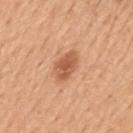Case summary:
* biopsy status — catalogued during a skin exam; not biopsied
* location — the mid back
* image — ~15 mm tile from a whole-body skin photo
* size — ~4 mm (longest diameter)
* automated metrics — an area of roughly 7 mm² and two-axis asymmetry of about 0.2; border irregularity of about 2 on a 0–10 scale, a color-variation rating of about 3/10, and peripheral color asymmetry of about 1; a detector confidence of about 100 out of 100 that the crop contains a lesion
* tile lighting — white-light
* subject — male, aged approximately 50